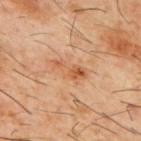This lesion was catalogued during total-body skin photography and was not selected for biopsy.
A male patient, approximately 60 years of age.
Approximately 4.5 mm at its widest.
This is a cross-polarized tile.
Located on the upper back.
A region of skin cropped from a whole-body photographic capture, roughly 15 mm wide.